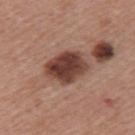Notes:
* workup · imaged on a skin check; not biopsied
* acquisition · 15 mm crop, total-body photography
* tile lighting · white-light
* lesion size · ≈5.5 mm
* subject · female, in their mid- to late 40s
* automated metrics · a mean CIELAB color near L≈41 a*≈21 b*≈25, a lesion–skin lightness drop of about 16, and a normalized border contrast of about 12
* location · the upper back